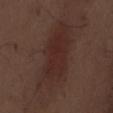{"patient": {"sex": "male", "age_approx": 70}, "image": {"source": "total-body photography crop", "field_of_view_mm": 15}, "site": "front of the torso"}